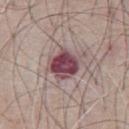Recorded during total-body skin imaging; not selected for excision or biopsy. Imaged with white-light lighting. Cropped from a total-body skin-imaging series; the visible field is about 15 mm. A male patient roughly 65 years of age. The lesion is located on the chest.